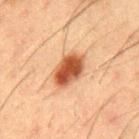biopsy_status: not biopsied; imaged during a skin examination